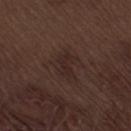Q: Is there a histopathology result?
A: total-body-photography surveillance lesion; no biopsy
Q: Patient demographics?
A: male, in their 70s
Q: What kind of image is this?
A: 15 mm crop, total-body photography
Q: Where on the body is the lesion?
A: the right thigh